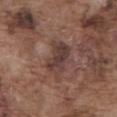  biopsy_status: not biopsied; imaged during a skin examination
  image:
    source: total-body photography crop
    field_of_view_mm: 15
  lighting: white-light
  patient:
    sex: male
    age_approx: 75
  site: abdomen